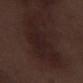Impression:
Captured during whole-body skin photography for melanoma surveillance; the lesion was not biopsied.
Background:
A roughly 15 mm field-of-view crop from a total-body skin photograph. Automated tile analysis of the lesion measured a lesion area of about 25 mm² and a shape-asymmetry score of about 0.45 (0 = symmetric). The analysis additionally found a lesion–skin lightness drop of about 5. The software also gave border irregularity of about 6.5 on a 0–10 scale. And it measured a nevus-likeness score of about 0/100. Measured at roughly 9.5 mm in maximum diameter. Located on the right lower leg. A male subject, roughly 70 years of age.